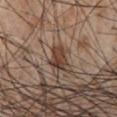Assessment:
Imaged during a routine full-body skin examination; the lesion was not biopsied and no histopathology is available.
Image and clinical context:
From the chest. A roughly 15 mm field-of-view crop from a total-body skin photograph. A male patient, aged 53 to 57.